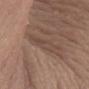Recorded during total-body skin imaging; not selected for excision or biopsy. This is a white-light tile. On the front of the torso. The lesion-visualizer software estimated a shape eccentricity near 0.85 and two-axis asymmetry of about 0.3. The software also gave a lesion–skin lightness drop of about 6 and a lesion-to-skin contrast of about 4.5 (normalized; higher = more distinct). It also reported a border-irregularity index near 4/10, a within-lesion color-variation index near 3/10, and a peripheral color-asymmetry measure near 1. And it measured a lesion-detection confidence of about 90/100. A 15 mm crop from a total-body photograph taken for skin-cancer surveillance. Approximately 5 mm at its widest. A female patient in their mid- to late 50s.